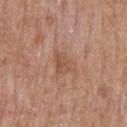On the back.
This is a white-light tile.
The patient is a male aged around 65.
The recorded lesion diameter is about 3.5 mm.
A region of skin cropped from a whole-body photographic capture, roughly 15 mm wide.